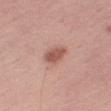Q: Was a biopsy performed?
A: no biopsy performed (imaged during a skin exam)
Q: Where on the body is the lesion?
A: the leg
Q: What kind of image is this?
A: total-body-photography crop, ~15 mm field of view
Q: Automated lesion metrics?
A: an area of roughly 5.5 mm² and a shape-asymmetry score of about 0.2 (0 = symmetric); a lesion–skin lightness drop of about 12 and a normalized lesion–skin contrast near 8; a border-irregularity index near 2/10, a within-lesion color-variation index near 2.5/10, and radial color variation of about 1; a classifier nevus-likeness of about 90/100 and lesion-presence confidence of about 100/100
Q: Who is the patient?
A: female, aged 43–47
Q: What lighting was used for the tile?
A: white-light illumination
Q: How large is the lesion?
A: about 3.5 mm